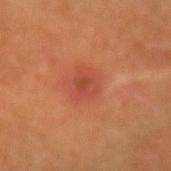| key | value |
|---|---|
| workup | imaged on a skin check; not biopsied |
| patient | female, aged approximately 50 |
| acquisition | ~15 mm tile from a whole-body skin photo |
| TBP lesion metrics | a footprint of about 5.5 mm², an eccentricity of roughly 0.55, and a symmetry-axis asymmetry near 0.3; a lesion color around L≈48 a*≈32 b*≈34 in CIELAB and a lesion-to-skin contrast of about 6 (normalized; higher = more distinct); a border-irregularity index near 2.5/10 and a peripheral color-asymmetry measure near 1.5 |